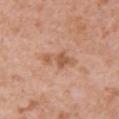workup: total-body-photography surveillance lesion; no biopsy
subject: female, about 40 years old
lighting: white-light illumination
image-analysis metrics: an average lesion color of about L≈57 a*≈23 b*≈33 (CIELAB), about 9 CIELAB-L* units darker than the surrounding skin, and a lesion-to-skin contrast of about 6.5 (normalized; higher = more distinct); a border-irregularity index near 4.5/10, a color-variation rating of about 2/10, and a peripheral color-asymmetry measure near 0.5; a lesion-detection confidence of about 100/100
diameter: ~4 mm (longest diameter)
image: ~15 mm tile from a whole-body skin photo
site: the right upper arm